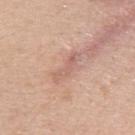<case>
<site>left upper arm</site>
<lesion_size>
  <long_diameter_mm_approx>5.0</long_diameter_mm_approx>
</lesion_size>
<image>
  <source>total-body photography crop</source>
  <field_of_view_mm>15</field_of_view_mm>
</image>
<patient>
  <sex>female</sex>
  <age_approx>45</age_approx>
</patient>
<automated_metrics>
  <cielab_L>62</cielab_L>
  <cielab_a>21</cielab_a>
  <cielab_b>26</cielab_b>
  <vs_skin_darker_L>8.0</vs_skin_darker_L>
  <vs_skin_contrast_norm>5.5</vs_skin_contrast_norm>
  <border_irregularity_0_10>5.5</border_irregularity_0_10>
  <peripheral_color_asymmetry>1.0</peripheral_color_asymmetry>
  <lesion_detection_confidence_0_100>55</lesion_detection_confidence_0_100>
</automated_metrics>
</case>